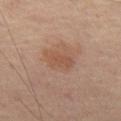notes = catalogued during a skin exam; not biopsied
patient = male, aged 63 to 67
lighting = cross-polarized illumination
automated lesion analysis = a lesion area of about 5.5 mm² and an eccentricity of roughly 0.85; a lesion-detection confidence of about 100/100
image = 15 mm crop, total-body photography
lesion diameter = about 3.5 mm
location = the left thigh